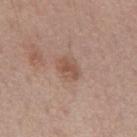Imaged during a routine full-body skin examination; the lesion was not biopsied and no histopathology is available.
This is a white-light tile.
The subject is a male aged 58–62.
The lesion is located on the mid back.
A 15 mm crop from a total-body photograph taken for skin-cancer surveillance.
The total-body-photography lesion software estimated an area of roughly 6 mm² and a shape-asymmetry score of about 0.2 (0 = symmetric). The analysis additionally found a mean CIELAB color near L≈53 a*≈19 b*≈27, a lesion–skin lightness drop of about 8, and a lesion-to-skin contrast of about 6 (normalized; higher = more distinct).